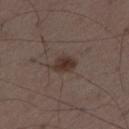Part of a total-body skin-imaging series; this lesion was reviewed on a skin check and was not flagged for biopsy.
A male patient aged approximately 50.
This is a white-light tile.
About 3 mm across.
A lesion tile, about 15 mm wide, cut from a 3D total-body photograph.
The lesion is located on the left thigh.